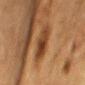workup = total-body-photography surveillance lesion; no biopsy | patient = female, in their mid- to late 50s | lesion diameter = ~5 mm (longest diameter) | location = the chest | lighting = cross-polarized illumination | image source = ~15 mm tile from a whole-body skin photo.